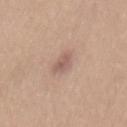Notes:
• biopsy status · imaged on a skin check; not biopsied
• patient · female, in their mid-30s
• body site · the mid back
• lesion diameter · ~3 mm (longest diameter)
• imaging modality · total-body-photography crop, ~15 mm field of view
• lighting · white-light